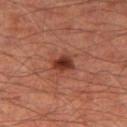Q: Was a biopsy performed?
A: total-body-photography surveillance lesion; no biopsy
Q: What is the lesion's diameter?
A: ≈2.5 mm
Q: Where on the body is the lesion?
A: the leg
Q: Automated lesion metrics?
A: a lesion area of about 4.5 mm² and an eccentricity of roughly 0.65; a border-irregularity index near 2.5/10, a color-variation rating of about 3/10, and peripheral color asymmetry of about 0.5; a classifier nevus-likeness of about 95/100 and lesion-presence confidence of about 100/100
Q: Patient demographics?
A: male, aged around 65
Q: What kind of image is this?
A: ~15 mm tile from a whole-body skin photo
Q: What lighting was used for the tile?
A: cross-polarized